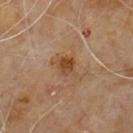Q: Was a biopsy performed?
A: imaged on a skin check; not biopsied
Q: Who is the patient?
A: male, approximately 60 years of age
Q: Automated lesion metrics?
A: a border-irregularity index near 3.5/10, a color-variation rating of about 5/10, and a peripheral color-asymmetry measure near 1.5
Q: Lesion location?
A: the upper back
Q: What is the imaging modality?
A: ~15 mm tile from a whole-body skin photo
Q: What is the lesion's diameter?
A: ≈3.5 mm
Q: Illumination type?
A: cross-polarized illumination